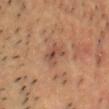{
  "biopsy_status": "not biopsied; imaged during a skin examination",
  "lighting": "cross-polarized",
  "patient": {
    "sex": "male",
    "age_approx": 40
  },
  "automated_metrics": {
    "area_mm2_approx": 4.5,
    "eccentricity": 0.7,
    "shape_asymmetry": 0.25,
    "cielab_L": 50,
    "cielab_a": 21,
    "cielab_b": 29
  },
  "site": "front of the torso",
  "image": {
    "source": "total-body photography crop",
    "field_of_view_mm": 15
  },
  "lesion_size": {
    "long_diameter_mm_approx": 2.5
  }
}